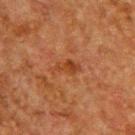No biopsy was performed on this lesion — it was imaged during a full skin examination and was not determined to be concerning. A male subject, aged 58–62. Cropped from a total-body skin-imaging series; the visible field is about 15 mm. Automated image analysis of the tile measured an eccentricity of roughly 0.85 and a shape-asymmetry score of about 0.3 (0 = symmetric). And it measured a border-irregularity index near 3.5/10, internal color variation of about 3 on a 0–10 scale, and peripheral color asymmetry of about 0.5. From the upper back. Longest diameter approximately 3.5 mm. This is a cross-polarized tile.